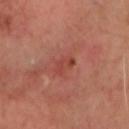– follow-up: catalogued during a skin exam; not biopsied
– image: total-body-photography crop, ~15 mm field of view
– illumination: cross-polarized
– body site: the head or neck
– subject: male, aged 68 to 72